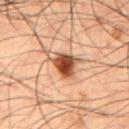Image and clinical context: The tile uses cross-polarized illumination. A male subject aged 48–52. Automated image analysis of the tile measured a lesion area of about 9 mm², an eccentricity of roughly 0.7, and a shape-asymmetry score of about 0.4 (0 = symmetric). And it measured an average lesion color of about L≈39 a*≈20 b*≈28 (CIELAB), roughly 15 lightness units darker than nearby skin, and a lesion-to-skin contrast of about 11.5 (normalized; higher = more distinct). And it measured a classifier nevus-likeness of about 100/100. A roughly 15 mm field-of-view crop from a total-body skin photograph. The lesion's longest dimension is about 4.5 mm. From the abdomen.